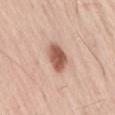notes = total-body-photography surveillance lesion; no biopsy
tile lighting = white-light illumination
site = the lower back
lesion size = ≈3.5 mm
image = ~15 mm crop, total-body skin-cancer survey
patient = male, in their mid-50s